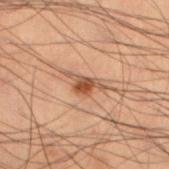| feature | finding |
|---|---|
| notes | no biopsy performed (imaged during a skin exam) |
| automated lesion analysis | a footprint of about 5 mm²; an average lesion color of about L≈43 a*≈19 b*≈28 (CIELAB); a classifier nevus-likeness of about 85/100 |
| subject | male, about 55 years old |
| tile lighting | cross-polarized illumination |
| anatomic site | the left lower leg |
| image | ~15 mm crop, total-body skin-cancer survey |
| size | ≈4 mm |